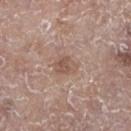The lesion was photographed on a routine skin check and not biopsied; there is no pathology result.
A female patient, aged 83 or older.
The recorded lesion diameter is about 3 mm.
Cropped from a whole-body photographic skin survey; the tile spans about 15 mm.
The lesion is located on the left lower leg.
The tile uses white-light illumination.